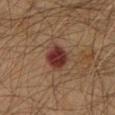A region of skin cropped from a whole-body photographic capture, roughly 15 mm wide. The lesion is on the upper back. The lesion's longest dimension is about 4 mm. A male subject, aged 58 to 62. Captured under cross-polarized illumination.